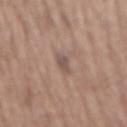Clinical impression:
The lesion was photographed on a routine skin check and not biopsied; there is no pathology result.
Context:
The subject is a female in their mid- to late 60s. The lesion-visualizer software estimated an outline eccentricity of about 0.9 (0 = round, 1 = elongated) and a shape-asymmetry score of about 0.2 (0 = symmetric). The software also gave a mean CIELAB color near L≈51 a*≈16 b*≈22, about 9 CIELAB-L* units darker than the surrounding skin, and a lesion-to-skin contrast of about 6.5 (normalized; higher = more distinct). And it measured border irregularity of about 2 on a 0–10 scale, internal color variation of about 0 on a 0–10 scale, and radial color variation of about 0. The analysis additionally found lesion-presence confidence of about 95/100. On the mid back. This is a white-light tile. A 15 mm close-up extracted from a 3D total-body photography capture. The recorded lesion diameter is about 2.5 mm.